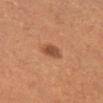Recorded during total-body skin imaging; not selected for excision or biopsy.
Approximately 3 mm at its widest.
Captured under cross-polarized illumination.
A male subject, aged 48 to 52.
The total-body-photography lesion software estimated an area of roughly 4.5 mm², an outline eccentricity of about 0.75 (0 = round, 1 = elongated), and a shape-asymmetry score of about 0.15 (0 = symmetric).
A close-up tile cropped from a whole-body skin photograph, about 15 mm across.
Located on the right lower leg.